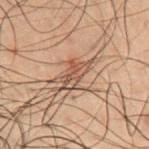Part of a total-body skin-imaging series; this lesion was reviewed on a skin check and was not flagged for biopsy.
About 4 mm across.
From the chest.
An algorithmic analysis of the crop reported a mean CIELAB color near L≈40 a*≈15 b*≈23. And it measured a border-irregularity rating of about 3.5/10 and internal color variation of about 5 on a 0–10 scale. The analysis additionally found an automated nevus-likeness rating near 5 out of 100 and a detector confidence of about 60 out of 100 that the crop contains a lesion.
Captured under cross-polarized illumination.
This image is a 15 mm lesion crop taken from a total-body photograph.
A male subject, about 50 years old.